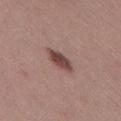<case>
  <lighting>white-light</lighting>
  <image>
    <source>total-body photography crop</source>
    <field_of_view_mm>15</field_of_view_mm>
  </image>
  <site>right thigh</site>
  <lesion_size>
    <long_diameter_mm_approx>4.0</long_diameter_mm_approx>
  </lesion_size>
  <automated_metrics>
    <vs_skin_darker_L>13.0</vs_skin_darker_L>
    <vs_skin_contrast_norm>10.0</vs_skin_contrast_norm>
    <border_irregularity_0_10>2.5</border_irregularity_0_10>
    <peripheral_color_asymmetry>1.0</peripheral_color_asymmetry>
    <nevus_likeness_0_100>100</nevus_likeness_0_100>
    <lesion_detection_confidence_0_100>100</lesion_detection_confidence_0_100>
  </automated_metrics>
  <patient>
    <sex>female</sex>
    <age_approx>45</age_approx>
  </patient>
</case>